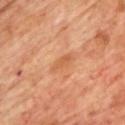Clinical impression:
Captured during whole-body skin photography for melanoma surveillance; the lesion was not biopsied.
Background:
A male patient, aged 68–72. Imaged with cross-polarized lighting. The lesion is located on the chest. Measured at roughly 3 mm in maximum diameter. Automated image analysis of the tile measured a classifier nevus-likeness of about 0/100 and lesion-presence confidence of about 100/100. A close-up tile cropped from a whole-body skin photograph, about 15 mm across.